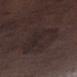subject = male, roughly 70 years of age | illumination = white-light illumination | anatomic site = the right lower leg | image source = 15 mm crop, total-body photography | lesion size = about 5 mm.